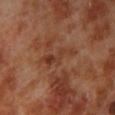Notes:
– follow-up — catalogued during a skin exam; not biopsied
– image — total-body-photography crop, ~15 mm field of view
– anatomic site — the left lower leg
– lesion diameter — about 4.5 mm
– subject — male, aged 68–72
– illumination — cross-polarized